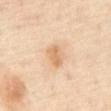| key | value |
|---|---|
| follow-up | no biopsy performed (imaged during a skin exam) |
| illumination | cross-polarized illumination |
| patient | male, in their mid-60s |
| image | ~15 mm tile from a whole-body skin photo |
| location | the abdomen |
| size | ~3 mm (longest diameter) |